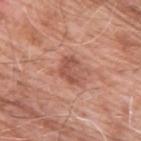follow-up: no biopsy performed (imaged during a skin exam) | image: ~15 mm crop, total-body skin-cancer survey | patient: male, about 60 years old | body site: the upper back.